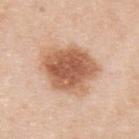Assessment:
Captured during whole-body skin photography for melanoma surveillance; the lesion was not biopsied.
Clinical summary:
This is a white-light tile. A male patient in their mid- to late 30s. A 15 mm close-up tile from a total-body photography series done for melanoma screening. The lesion is on the upper back. The lesion's longest dimension is about 7 mm. Automated image analysis of the tile measured a footprint of about 27 mm², an eccentricity of roughly 0.6, and a shape-asymmetry score of about 0.2 (0 = symmetric). The analysis additionally found lesion-presence confidence of about 100/100.